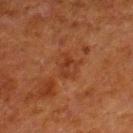Imaged during a routine full-body skin examination; the lesion was not biopsied and no histopathology is available.